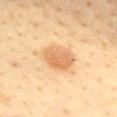imaging modality = ~15 mm crop, total-body skin-cancer survey; diameter = ≈4 mm; subject = female, aged approximately 50; site = the chest.